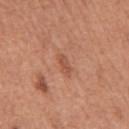The lesion was photographed on a routine skin check and not biopsied; there is no pathology result.
An algorithmic analysis of the crop reported a lesion area of about 3 mm², an outline eccentricity of about 0.85 (0 = round, 1 = elongated), and two-axis asymmetry of about 0.25. The software also gave a mean CIELAB color near L≈53 a*≈25 b*≈33, about 8 CIELAB-L* units darker than the surrounding skin, and a normalized border contrast of about 5.5. The software also gave border irregularity of about 2.5 on a 0–10 scale and a peripheral color-asymmetry measure near 0.5. And it measured an automated nevus-likeness rating near 0 out of 100.
This image is a 15 mm lesion crop taken from a total-body photograph.
On the mid back.
A male subject, in their mid- to late 60s.
This is a white-light tile.